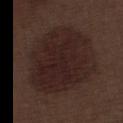Recorded during total-body skin imaging; not selected for excision or biopsy. Located on the left thigh. The patient is a male in their 70s. The recorded lesion diameter is about 9 mm. A region of skin cropped from a whole-body photographic capture, roughly 15 mm wide. Captured under white-light illumination.